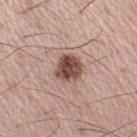Clinical summary:
A 15 mm crop from a total-body photograph taken for skin-cancer surveillance. Imaged with white-light lighting. The lesion is located on the right upper arm. Longest diameter approximately 3.5 mm. A male subject, approximately 55 years of age.